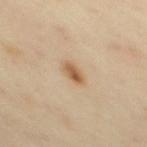This lesion was catalogued during total-body skin photography and was not selected for biopsy.
A female patient approximately 40 years of age.
A 15 mm crop from a total-body photograph taken for skin-cancer surveillance.
The lesion is located on the upper back.